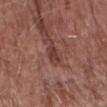The lesion was tiled from a total-body skin photograph and was not biopsied.
Captured under white-light illumination.
The total-body-photography lesion software estimated a lesion area of about 3 mm², an outline eccentricity of about 0.9 (0 = round, 1 = elongated), and a symmetry-axis asymmetry near 0.35. It also reported a classifier nevus-likeness of about 5/100.
Longest diameter approximately 2.5 mm.
The lesion is on the head or neck.
A male patient, roughly 80 years of age.
Cropped from a total-body skin-imaging series; the visible field is about 15 mm.